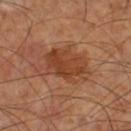Q: Is there a histopathology result?
A: no biopsy performed (imaged during a skin exam)
Q: What is the anatomic site?
A: the right thigh
Q: Lesion size?
A: about 6.5 mm
Q: What kind of image is this?
A: 15 mm crop, total-body photography
Q: Patient demographics?
A: male, aged approximately 60
Q: How was the tile lit?
A: cross-polarized
Q: Automated lesion metrics?
A: about 9 CIELAB-L* units darker than the surrounding skin and a normalized border contrast of about 7.5; peripheral color asymmetry of about 1.5; a classifier nevus-likeness of about 25/100 and lesion-presence confidence of about 100/100